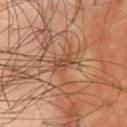Assessment:
No biopsy was performed on this lesion — it was imaged during a full skin examination and was not determined to be concerning.
Clinical summary:
This is a cross-polarized tile. A male subject, in their mid-40s. A 15 mm crop from a total-body photograph taken for skin-cancer surveillance. On the front of the torso. Automated image analysis of the tile measured a lesion area of about 4 mm², an eccentricity of roughly 0.85, and a symmetry-axis asymmetry near 0.4. It also reported peripheral color asymmetry of about 0.5.